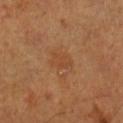workup = imaged on a skin check; not biopsied
lesion size = ~3 mm (longest diameter)
location = the right lower leg
patient = male, aged approximately 55
imaging modality = ~15 mm crop, total-body skin-cancer survey